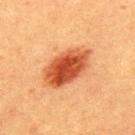Recorded during total-body skin imaging; not selected for excision or biopsy. Located on the upper back. Measured at roughly 6 mm in maximum diameter. The total-body-photography lesion software estimated a footprint of about 17 mm² and a shape-asymmetry score of about 0.1 (0 = symmetric). And it measured a lesion color around L≈44 a*≈29 b*≈36 in CIELAB and a lesion–skin lightness drop of about 15. The software also gave a detector confidence of about 100 out of 100 that the crop contains a lesion. A male subject aged approximately 40. A 15 mm close-up extracted from a 3D total-body photography capture.